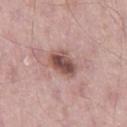Assessment:
No biopsy was performed on this lesion — it was imaged during a full skin examination and was not determined to be concerning.
Acquisition and patient details:
Captured under white-light illumination. The subject is a male about 55 years old. The lesion is located on the right thigh. A roughly 15 mm field-of-view crop from a total-body skin photograph.